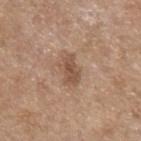Image and clinical context:
Measured at roughly 3.5 mm in maximum diameter. An algorithmic analysis of the crop reported an area of roughly 5.5 mm², an eccentricity of roughly 0.8, and two-axis asymmetry of about 0.3. It also reported a border-irregularity rating of about 3.5/10, internal color variation of about 2.5 on a 0–10 scale, and radial color variation of about 1. The software also gave a nevus-likeness score of about 15/100. Located on the left forearm. A female subject roughly 65 years of age. A close-up tile cropped from a whole-body skin photograph, about 15 mm across. Captured under white-light illumination.